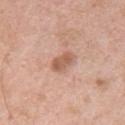| field | value |
|---|---|
| notes | imaged on a skin check; not biopsied |
| body site | the chest |
| illumination | white-light |
| acquisition | total-body-photography crop, ~15 mm field of view |
| lesion diameter | ~3 mm (longest diameter) |
| TBP lesion metrics | an area of roughly 5 mm², an outline eccentricity of about 0.8 (0 = round, 1 = elongated), and a symmetry-axis asymmetry near 0.2; roughly 11 lightness units darker than nearby skin and a normalized lesion–skin contrast near 7.5; a border-irregularity rating of about 2/10 and peripheral color asymmetry of about 1 |
| subject | male, approximately 55 years of age |